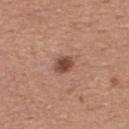body site = the back
lesion size = ~2.5 mm (longest diameter)
image = ~15 mm crop, total-body skin-cancer survey
subject = female, about 35 years old
lighting = white-light illumination
automated metrics = a lesion color around L≈47 a*≈23 b*≈27 in CIELAB, roughly 13 lightness units darker than nearby skin, and a normalized border contrast of about 9.5